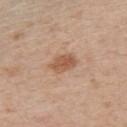Recorded during total-body skin imaging; not selected for excision or biopsy.
From the arm.
Automated image analysis of the tile measured a border-irregularity index near 2/10 and a within-lesion color-variation index near 2.5/10. And it measured an automated nevus-likeness rating near 80 out of 100.
The subject is a male in their mid-50s.
The tile uses white-light illumination.
A region of skin cropped from a whole-body photographic capture, roughly 15 mm wide.
Longest diameter approximately 3 mm.